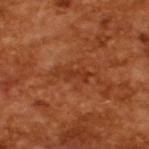The lesion was tiled from a total-body skin photograph and was not biopsied. Measured at roughly 4.5 mm in maximum diameter. A male patient, approximately 65 years of age. A 15 mm crop from a total-body photograph taken for skin-cancer surveillance.